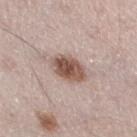The lesion was photographed on a routine skin check and not biopsied; there is no pathology result.